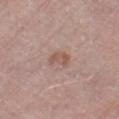{
  "image": {
    "source": "total-body photography crop",
    "field_of_view_mm": 15
  },
  "patient": {
    "sex": "male",
    "age_approx": 80
  },
  "site": "right thigh",
  "lesion_size": {
    "long_diameter_mm_approx": 3.0
  }
}